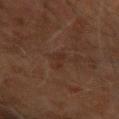Captured during whole-body skin photography for melanoma surveillance; the lesion was not biopsied. Automated image analysis of the tile measured an area of roughly 2.5 mm², a shape eccentricity near 0.9, and a symmetry-axis asymmetry near 0.5. The analysis additionally found a lesion–skin lightness drop of about 4 and a normalized lesion–skin contrast near 4.5. The analysis additionally found a color-variation rating of about 0/10 and radial color variation of about 0. The analysis additionally found a nevus-likeness score of about 0/100 and a detector confidence of about 100 out of 100 that the crop contains a lesion. A male patient aged 68 to 72. Imaged with cross-polarized lighting. The recorded lesion diameter is about 3 mm. The lesion is located on the right upper arm. Cropped from a total-body skin-imaging series; the visible field is about 15 mm.